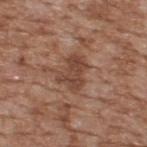Acquisition and patient details: A 15 mm close-up tile from a total-body photography series done for melanoma screening. Automated tile analysis of the lesion measured a lesion color around L≈44 a*≈20 b*≈28 in CIELAB, a lesion–skin lightness drop of about 9, and a normalized lesion–skin contrast near 7.5. From the upper back. Measured at roughly 4 mm in maximum diameter. The subject is a male roughly 65 years of age.